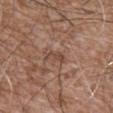<lesion>
<biopsy_status>not biopsied; imaged during a skin examination</biopsy_status>
<patient>
  <sex>male</sex>
  <age_approx>75</age_approx>
</patient>
<image>
  <source>total-body photography crop</source>
  <field_of_view_mm>15</field_of_view_mm>
</image>
<site>abdomen</site>
</lesion>